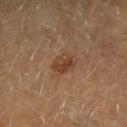The lesion was tiled from a total-body skin photograph and was not biopsied.